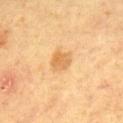biopsy status: no biopsy performed (imaged during a skin exam) | tile lighting: cross-polarized illumination | image: 15 mm crop, total-body photography | subject: male, approximately 65 years of age | body site: the upper back | size: about 3 mm | automated lesion analysis: a lesion area of about 5.5 mm², an outline eccentricity of about 0.65 (0 = round, 1 = elongated), and a symmetry-axis asymmetry near 0.2; a mean CIELAB color near L≈61 a*≈20 b*≈42; a within-lesion color-variation index near 3/10 and a peripheral color-asymmetry measure near 1; a nevus-likeness score of about 45/100 and lesion-presence confidence of about 100/100.